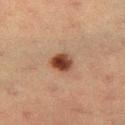This lesion was catalogued during total-body skin photography and was not selected for biopsy. Cropped from a total-body skin-imaging series; the visible field is about 15 mm. A female patient, roughly 55 years of age. The lesion is located on the left thigh.